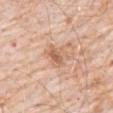Impression:
No biopsy was performed on this lesion — it was imaged during a full skin examination and was not determined to be concerning.
Background:
About 3 mm across. The subject is a male aged around 80. The lesion is located on the abdomen. A region of skin cropped from a whole-body photographic capture, roughly 15 mm wide. The tile uses white-light illumination.